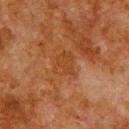The lesion was photographed on a routine skin check and not biopsied; there is no pathology result.
The lesion is on the upper back.
Longest diameter approximately 3 mm.
A male subject roughly 80 years of age.
A lesion tile, about 15 mm wide, cut from a 3D total-body photograph.
This is a cross-polarized tile.
The lesion-visualizer software estimated an outline eccentricity of about 0.6 (0 = round, 1 = elongated) and a symmetry-axis asymmetry near 0.25. The software also gave an average lesion color of about L≈34 a*≈21 b*≈32 (CIELAB), roughly 4 lightness units darker than nearby skin, and a lesion-to-skin contrast of about 4.5 (normalized; higher = more distinct). The software also gave a border-irregularity index near 2.5/10 and internal color variation of about 1 on a 0–10 scale.